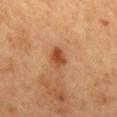Captured during whole-body skin photography for melanoma surveillance; the lesion was not biopsied.
A female subject, in their 60s.
Imaged with cross-polarized lighting.
Cropped from a total-body skin-imaging series; the visible field is about 15 mm.
The lesion is located on the mid back.
Approximately 3 mm at its widest.